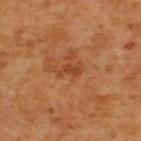| field | value |
|---|---|
| notes | catalogued during a skin exam; not biopsied |
| tile lighting | cross-polarized |
| location | the upper back |
| automated lesion analysis | a nevus-likeness score of about 0/100 and a detector confidence of about 100 out of 100 that the crop contains a lesion |
| image source | ~15 mm crop, total-body skin-cancer survey |
| subject | male, aged 63 to 67 |
| diameter | ≈2.5 mm |